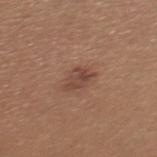Impression:
Captured during whole-body skin photography for melanoma surveillance; the lesion was not biopsied.
Context:
The lesion is located on the mid back. Longest diameter approximately 3 mm. A 15 mm close-up tile from a total-body photography series done for melanoma screening. The subject is a female aged approximately 25.